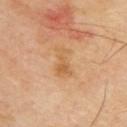{"biopsy_status": "not biopsied; imaged during a skin examination", "image": {"source": "total-body photography crop", "field_of_view_mm": 15}, "patient": {"sex": "male", "age_approx": 65}, "site": "upper back"}